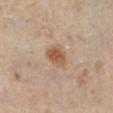workup = catalogued during a skin exam; not biopsied
image = ~15 mm tile from a whole-body skin photo
lesion size = ~3 mm (longest diameter)
image-analysis metrics = a lesion area of about 6 mm² and two-axis asymmetry of about 0.2; a border-irregularity rating of about 2/10 and a color-variation rating of about 3/10
illumination = cross-polarized
subject = female, approximately 60 years of age
location = the leg